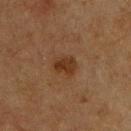Q: Was this lesion biopsied?
A: catalogued during a skin exam; not biopsied
Q: How was the tile lit?
A: cross-polarized illumination
Q: What are the patient's age and sex?
A: male, aged 73 to 77
Q: Automated lesion metrics?
A: a mean CIELAB color near L≈27 a*≈17 b*≈26 and a normalized border contrast of about 8
Q: Lesion location?
A: the chest
Q: What is the imaging modality?
A: total-body-photography crop, ~15 mm field of view